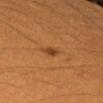Assessment:
Part of a total-body skin-imaging series; this lesion was reviewed on a skin check and was not flagged for biopsy.
Context:
Measured at roughly 2 mm in maximum diameter. From the left forearm. An algorithmic analysis of the crop reported an average lesion color of about L≈42 a*≈25 b*≈38 (CIELAB) and a lesion–skin lightness drop of about 10. A female subject in their 40s. A 15 mm crop from a total-body photograph taken for skin-cancer surveillance.